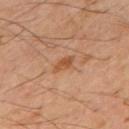Notes:
- follow-up: total-body-photography surveillance lesion; no biopsy
- lesion diameter: ≈3 mm
- site: the mid back
- subject: male, in their mid- to late 40s
- imaging modality: 15 mm crop, total-body photography
- illumination: cross-polarized
- automated metrics: an average lesion color of about L≈49 a*≈22 b*≈35 (CIELAB) and a normalized lesion–skin contrast near 7; border irregularity of about 3 on a 0–10 scale, internal color variation of about 2 on a 0–10 scale, and a peripheral color-asymmetry measure near 0.5